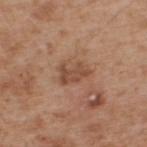follow-up: total-body-photography surveillance lesion; no biopsy
image source: 15 mm crop, total-body photography
image-analysis metrics: an eccentricity of roughly 0.8 and a symmetry-axis asymmetry near 0.45; a lesion color around L≈48 a*≈21 b*≈31 in CIELAB, about 9 CIELAB-L* units darker than the surrounding skin, and a normalized border contrast of about 7; a border-irregularity rating of about 5/10, a color-variation rating of about 2.5/10, and peripheral color asymmetry of about 1
subject: male, aged 63–67
location: the back
diameter: ≈3.5 mm
tile lighting: white-light illumination